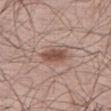follow-up = no biopsy performed (imaged during a skin exam) | site = the leg | patient = male, in their 60s | acquisition = ~15 mm crop, total-body skin-cancer survey | TBP lesion metrics = a footprint of about 6 mm², an eccentricity of roughly 0.75, and a symmetry-axis asymmetry near 0.2; a lesion color around L≈51 a*≈20 b*≈25 in CIELAB, about 11 CIELAB-L* units darker than the surrounding skin, and a normalized lesion–skin contrast near 8.5 | lesion diameter = ~3.5 mm (longest diameter).